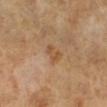Acquisition and patient details: Automated image analysis of the tile measured a lesion area of about 4.5 mm², an outline eccentricity of about 0.8 (0 = round, 1 = elongated), and a shape-asymmetry score of about 0.35 (0 = symmetric). And it measured an average lesion color of about L≈53 a*≈19 b*≈36 (CIELAB), roughly 7 lightness units darker than nearby skin, and a normalized border contrast of about 6. It also reported border irregularity of about 3.5 on a 0–10 scale, a color-variation rating of about 2.5/10, and a peripheral color-asymmetry measure near 0.5. The analysis additionally found lesion-presence confidence of about 100/100. Captured under cross-polarized illumination. On the right lower leg. The subject is a female approximately 55 years of age. A region of skin cropped from a whole-body photographic capture, roughly 15 mm wide.